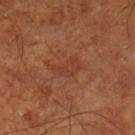Q: Where on the body is the lesion?
A: the left lower leg
Q: What did automated image analysis measure?
A: a footprint of about 6 mm² and a symmetry-axis asymmetry near 0.5; a border-irregularity index near 5/10 and radial color variation of about 0.5; a nevus-likeness score of about 0/100 and a detector confidence of about 100 out of 100 that the crop contains a lesion
Q: Patient demographics?
A: in their mid- to late 60s
Q: What is the lesion's diameter?
A: ~4 mm (longest diameter)
Q: What is the imaging modality?
A: total-body-photography crop, ~15 mm field of view